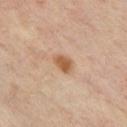  biopsy_status: not biopsied; imaged during a skin examination
  lighting: cross-polarized
  patient:
    sex: male
    age_approx: 45
  site: front of the torso
  lesion_size:
    long_diameter_mm_approx: 2.5
  automated_metrics:
    area_mm2_approx: 4.0
    eccentricity: 0.75
    shape_asymmetry: 0.2
    cielab_L: 55
    cielab_a: 20
    cielab_b: 34
    vs_skin_darker_L: 11.0
    vs_skin_contrast_norm: 8.5
    border_irregularity_0_10: 2.0
    color_variation_0_10: 2.5
    peripheral_color_asymmetry: 1.0
  image:
    source: total-body photography crop
    field_of_view_mm: 15